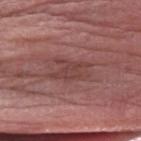Clinical impression:
Recorded during total-body skin imaging; not selected for excision or biopsy.
Clinical summary:
This image is a 15 mm lesion crop taken from a total-body photograph. A male patient, in their 80s. From the left forearm.